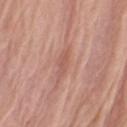Q: Was this lesion biopsied?
A: no biopsy performed (imaged during a skin exam)
Q: Where on the body is the lesion?
A: the left upper arm
Q: What are the patient's age and sex?
A: female, roughly 80 years of age
Q: What kind of image is this?
A: ~15 mm tile from a whole-body skin photo